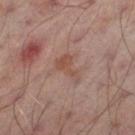<lesion>
  <biopsy_status>not biopsied; imaged during a skin examination</biopsy_status>
  <site>left thigh</site>
  <lesion_size>
    <long_diameter_mm_approx>3.5</long_diameter_mm_approx>
  </lesion_size>
  <patient>
    <sex>male</sex>
    <age_approx>65</age_approx>
  </patient>
  <image>
    <source>total-body photography crop</source>
    <field_of_view_mm>15</field_of_view_mm>
  </image>
  <lighting>cross-polarized</lighting>
  <automated_metrics>
    <area_mm2_approx>5.0</area_mm2_approx>
    <eccentricity>0.85</eccentricity>
    <shape_asymmetry>0.55</shape_asymmetry>
    <nevus_likeness_0_100>0</nevus_likeness_0_100>
    <lesion_detection_confidence_0_100>100</lesion_detection_confidence_0_100>
  </automated_metrics>
</lesion>